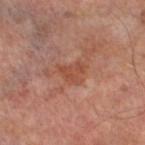Clinical impression:
Part of a total-body skin-imaging series; this lesion was reviewed on a skin check and was not flagged for biopsy.
Context:
Longest diameter approximately 3 mm. The lesion is located on the left thigh. A male patient, roughly 65 years of age. The tile uses cross-polarized illumination. A close-up tile cropped from a whole-body skin photograph, about 15 mm across.